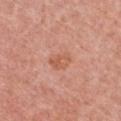Clinical impression:
No biopsy was performed on this lesion — it was imaged during a full skin examination and was not determined to be concerning.
Image and clinical context:
A 15 mm close-up extracted from a 3D total-body photography capture. Captured under white-light illumination. From the front of the torso. The patient is a female aged approximately 60.